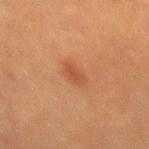<case>
<biopsy_status>not biopsied; imaged during a skin examination</biopsy_status>
<lighting>cross-polarized</lighting>
<patient>
  <sex>male</sex>
  <age_approx>50</age_approx>
</patient>
<site>mid back</site>
<image>
  <source>total-body photography crop</source>
  <field_of_view_mm>15</field_of_view_mm>
</image>
</case>